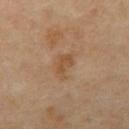No biopsy was performed on this lesion — it was imaged during a full skin examination and was not determined to be concerning. The tile uses cross-polarized illumination. On the abdomen. A male patient, aged around 65. A lesion tile, about 15 mm wide, cut from a 3D total-body photograph. About 3.5 mm across.